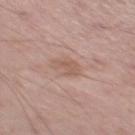  biopsy_status: not biopsied; imaged during a skin examination
  lesion_size:
    long_diameter_mm_approx: 3.0
  site: left thigh
  lighting: white-light
  image:
    source: total-body photography crop
    field_of_view_mm: 15
  automated_metrics:
    nevus_likeness_0_100: 0
    lesion_detection_confidence_0_100: 100
  patient:
    sex: male
    age_approx: 55